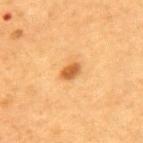notes = imaged on a skin check; not biopsied
patient = female, aged 58–62
image source = ~15 mm crop, total-body skin-cancer survey
automated lesion analysis = a lesion area of about 3.5 mm², an eccentricity of roughly 0.8, and a symmetry-axis asymmetry near 0.3; a lesion color around L≈49 a*≈22 b*≈40 in CIELAB, roughly 12 lightness units darker than nearby skin, and a normalized border contrast of about 8.5; a border-irregularity rating of about 2.5/10, a color-variation rating of about 2.5/10, and peripheral color asymmetry of about 1
lesion diameter = ~2.5 mm (longest diameter)
body site = the back